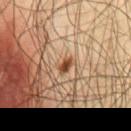Clinical impression:
The lesion was photographed on a routine skin check and not biopsied; there is no pathology result.
Clinical summary:
A male patient in their mid-60s. Approximately 2.5 mm at its widest. The lesion is located on the chest. Captured under cross-polarized illumination. A 15 mm close-up tile from a total-body photography series done for melanoma screening.